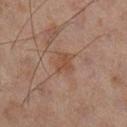This lesion was catalogued during total-body skin photography and was not selected for biopsy. Located on the right lower leg. Approximately 2.5 mm at its widest. The subject is a male about 45 years old. A close-up tile cropped from a whole-body skin photograph, about 15 mm across. Captured under cross-polarized illumination. An algorithmic analysis of the crop reported an average lesion color of about L≈37 a*≈17 b*≈24 (CIELAB), about 5 CIELAB-L* units darker than the surrounding skin, and a lesion-to-skin contrast of about 6 (normalized; higher = more distinct). The software also gave radial color variation of about 0.5.